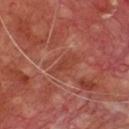Case summary:
- notes: total-body-photography surveillance lesion; no biopsy
- automated metrics: a lesion area of about 5.5 mm² and a symmetry-axis asymmetry near 0.25; an automated nevus-likeness rating near 0 out of 100 and a detector confidence of about 65 out of 100 that the crop contains a lesion
- tile lighting: cross-polarized
- imaging modality: ~15 mm crop, total-body skin-cancer survey
- subject: male, about 70 years old
- body site: the chest
- lesion diameter: about 3.5 mm